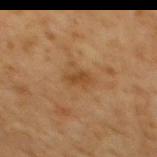Impression: This lesion was catalogued during total-body skin photography and was not selected for biopsy. Image and clinical context: Located on the mid back. A 15 mm close-up extracted from a 3D total-body photography capture. The patient is a male roughly 60 years of age.